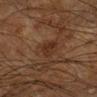<lesion>
<biopsy_status>not biopsied; imaged during a skin examination</biopsy_status>
<patient>
  <sex>male</sex>
  <age_approx>65</age_approx>
</patient>
<site>right lower leg</site>
<image>
  <source>total-body photography crop</source>
  <field_of_view_mm>15</field_of_view_mm>
</image>
<lesion_size>
  <long_diameter_mm_approx>2.5</long_diameter_mm_approx>
</lesion_size>
</lesion>